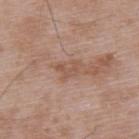Assessment:
No biopsy was performed on this lesion — it was imaged during a full skin examination and was not determined to be concerning.